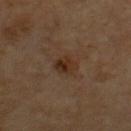Q: What is the imaging modality?
A: ~15 mm crop, total-body skin-cancer survey
Q: What is the lesion's diameter?
A: about 3 mm
Q: Who is the patient?
A: male, roughly 60 years of age
Q: Lesion location?
A: the chest